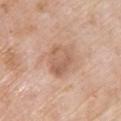This lesion was catalogued during total-body skin photography and was not selected for biopsy. Measured at roughly 4 mm in maximum diameter. The tile uses white-light illumination. A female subject, aged approximately 70. Located on the upper back. The lesion-visualizer software estimated an area of roughly 10 mm² and two-axis asymmetry of about 0.2. The analysis additionally found a mean CIELAB color near L≈60 a*≈20 b*≈30 and roughly 9 lightness units darker than nearby skin. A 15 mm crop from a total-body photograph taken for skin-cancer surveillance.